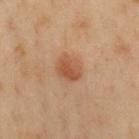workup: catalogued during a skin exam; not biopsied | acquisition: 15 mm crop, total-body photography | anatomic site: the chest | subject: male, approximately 55 years of age | lesion diameter: about 3 mm.